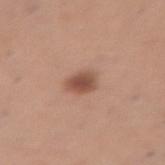Assessment:
Imaged during a routine full-body skin examination; the lesion was not biopsied and no histopathology is available.
Background:
A lesion tile, about 15 mm wide, cut from a 3D total-body photograph. Captured under white-light illumination. The subject is a female aged 48–52. The lesion is located on the abdomen. Longest diameter approximately 3 mm. The lesion-visualizer software estimated an area of roughly 5.5 mm², an eccentricity of roughly 0.7, and two-axis asymmetry of about 0.2. And it measured a classifier nevus-likeness of about 100/100 and a lesion-detection confidence of about 100/100.